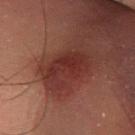notes = imaged on a skin check; not biopsied | subject = male, aged approximately 50 | lesion diameter = about 5 mm | site = the left lower leg | tile lighting = cross-polarized illumination | TBP lesion metrics = an area of roughly 13 mm², an eccentricity of roughly 0.7, and a symmetry-axis asymmetry near 0.25; border irregularity of about 4 on a 0–10 scale and peripheral color asymmetry of about 1; a classifier nevus-likeness of about 0/100 and a detector confidence of about 95 out of 100 that the crop contains a lesion | image = 15 mm crop, total-body photography.